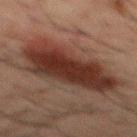Recorded during total-body skin imaging; not selected for excision or biopsy.
An algorithmic analysis of the crop reported an area of roughly 32 mm², an eccentricity of roughly 0.9, and a symmetry-axis asymmetry near 0.25. The software also gave a border-irregularity index near 3.5/10, a color-variation rating of about 4.5/10, and a peripheral color-asymmetry measure near 1.5.
The tile uses cross-polarized illumination.
A male subject, roughly 50 years of age.
From the back.
A roughly 15 mm field-of-view crop from a total-body skin photograph.